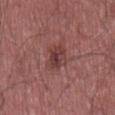No biopsy was performed on this lesion — it was imaged during a full skin examination and was not determined to be concerning. Located on the lower back. The patient is a male aged 48 to 52. Cropped from a whole-body photographic skin survey; the tile spans about 15 mm. Imaged with white-light lighting.